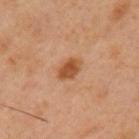follow-up — total-body-photography surveillance lesion; no biopsy | subject — male, in their mid-40s | location — the left upper arm | image source — total-body-photography crop, ~15 mm field of view.